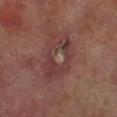The total-body-photography lesion software estimated an area of roughly 17 mm² and a symmetry-axis asymmetry near 0.2. And it measured a classifier nevus-likeness of about 0/100.
From the right lower leg.
The patient is a male roughly 70 years of age.
Captured under cross-polarized illumination.
A 15 mm crop from a total-body photograph taken for skin-cancer surveillance.